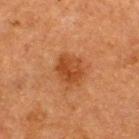Imaged during a routine full-body skin examination; the lesion was not biopsied and no histopathology is available. The lesion is located on the upper back. A male subject in their 50s. Cropped from a whole-body photographic skin survey; the tile spans about 15 mm.